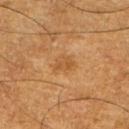Findings:
• biopsy status: imaged on a skin check; not biopsied
• patient: male, in their mid-50s
• image source: total-body-photography crop, ~15 mm field of view
• tile lighting: cross-polarized
• size: ~3 mm (longest diameter)
• location: the right upper arm
• image-analysis metrics: an area of roughly 5 mm², a shape eccentricity near 0.65, and two-axis asymmetry of about 0.2; a border-irregularity index near 2.5/10, internal color variation of about 2 on a 0–10 scale, and radial color variation of about 0.5